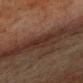body site: the back; illumination: cross-polarized illumination; image: ~15 mm crop, total-body skin-cancer survey; lesion size: ~3 mm (longest diameter); patient: male, roughly 75 years of age; automated metrics: an eccentricity of roughly 0.65 and a shape-asymmetry score of about 0.45 (0 = symmetric).